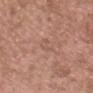Part of a total-body skin-imaging series; this lesion was reviewed on a skin check and was not flagged for biopsy. From the head or neck. Longest diameter approximately 3 mm. Imaged with white-light lighting. The subject is a male aged around 50. A 15 mm close-up extracted from a 3D total-body photography capture.